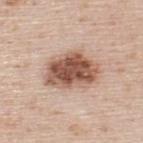Assessment: Recorded during total-body skin imaging; not selected for excision or biopsy. Clinical summary: A 15 mm close-up tile from a total-body photography series done for melanoma screening. Imaged with white-light lighting. A male patient, aged 43–47. Located on the upper back.